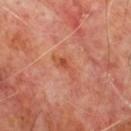Acquisition and patient details:
The tile uses cross-polarized illumination. A roughly 15 mm field-of-view crop from a total-body skin photograph. About 3.5 mm across. The lesion is on the chest. A male subject, aged around 65.